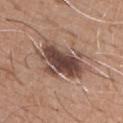Findings:
* workup: imaged on a skin check; not biopsied
* size: about 6 mm
* acquisition: 15 mm crop, total-body photography
* site: the chest
* subject: male, in their mid- to late 60s